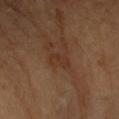Clinical impression: No biopsy was performed on this lesion — it was imaged during a full skin examination and was not determined to be concerning. Context: This image is a 15 mm lesion crop taken from a total-body photograph. The total-body-photography lesion software estimated an outline eccentricity of about 0.8 (0 = round, 1 = elongated) and a shape-asymmetry score of about 0.4 (0 = symmetric). The software also gave a classifier nevus-likeness of about 0/100 and lesion-presence confidence of about 100/100. Imaged with cross-polarized lighting. A female patient, aged approximately 70. From the left forearm.